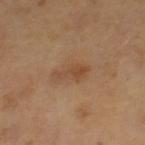The lesion was photographed on a routine skin check and not biopsied; there is no pathology result. A close-up tile cropped from a whole-body skin photograph, about 15 mm across. Captured under cross-polarized illumination. The patient is a female aged 53 to 57. Measured at roughly 3.5 mm in maximum diameter. The lesion is on the left thigh.